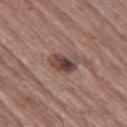<tbp_lesion>
<image>
  <source>total-body photography crop</source>
  <field_of_view_mm>15</field_of_view_mm>
</image>
<site>left thigh</site>
<patient>
  <sex>male</sex>
  <age_approx>65</age_approx>
</patient>
</tbp_lesion>